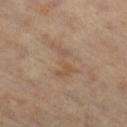biopsy status — no biopsy performed (imaged during a skin exam) | lighting — cross-polarized | image — ~15 mm tile from a whole-body skin photo | subject — male, approximately 60 years of age | anatomic site — the right thigh.